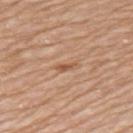Assessment: The lesion was tiled from a total-body skin photograph and was not biopsied. Context: A female subject, approximately 75 years of age. On the upper back. This image is a 15 mm lesion crop taken from a total-body photograph. Measured at roughly 3 mm in maximum diameter.